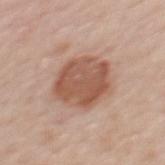No biopsy was performed on this lesion — it was imaged during a full skin examination and was not determined to be concerning.